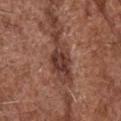follow-up: no biopsy performed (imaged during a skin exam) | automated lesion analysis: a lesion color around L≈38 a*≈22 b*≈26 in CIELAB and a lesion–skin lightness drop of about 11; border irregularity of about 6 on a 0–10 scale and peripheral color asymmetry of about 1.5 | size: ≈6.5 mm | illumination: white-light illumination | patient: male, in their mid-70s | body site: the head or neck | image source: ~15 mm crop, total-body skin-cancer survey.